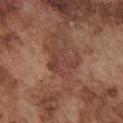A close-up tile cropped from a whole-body skin photograph, about 15 mm across. On the chest. The total-body-photography lesion software estimated an area of roughly 6 mm² and a shape-asymmetry score of about 0.7 (0 = symmetric). It also reported a lesion color around L≈41 a*≈23 b*≈25 in CIELAB, a lesion–skin lightness drop of about 7, and a normalized lesion–skin contrast near 6. And it measured a border-irregularity index near 8.5/10, a within-lesion color-variation index near 3/10, and a peripheral color-asymmetry measure near 1. About 4 mm across. A male patient, about 75 years old.